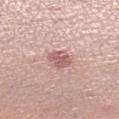<lesion>
  <biopsy_status>not biopsied; imaged during a skin examination</biopsy_status>
  <image>
    <source>total-body photography crop</source>
    <field_of_view_mm>15</field_of_view_mm>
  </image>
  <patient>
    <sex>female</sex>
    <age_approx>55</age_approx>
  </patient>
  <lesion_size>
    <long_diameter_mm_approx>2.5</long_diameter_mm_approx>
  </lesion_size>
  <site>leg</site>
  <lighting>white-light</lighting>
</lesion>